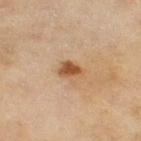The subject is a female aged around 60. A 15 mm crop from a total-body photograph taken for skin-cancer surveillance. The lesion is located on the right thigh.